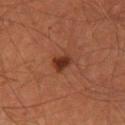Assessment: The lesion was photographed on a routine skin check and not biopsied; there is no pathology result. Background: Captured under cross-polarized illumination. A male subject, aged 63 to 67. The lesion is located on the right thigh. A lesion tile, about 15 mm wide, cut from a 3D total-body photograph.